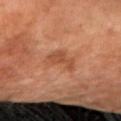The subject is a female roughly 70 years of age.
Imaged with cross-polarized lighting.
The lesion is located on the right forearm.
A close-up tile cropped from a whole-body skin photograph, about 15 mm across.